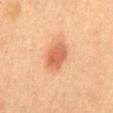biopsy status: no biopsy performed (imaged during a skin exam) | patient: female, in their 60s | acquisition: ~15 mm tile from a whole-body skin photo | tile lighting: cross-polarized illumination | anatomic site: the mid back | diameter: ~4 mm (longest diameter) | TBP lesion metrics: a lesion area of about 8.5 mm², an eccentricity of roughly 0.8, and a shape-asymmetry score of about 0.25 (0 = symmetric); lesion-presence confidence of about 100/100.